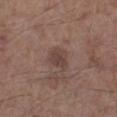No biopsy was performed on this lesion — it was imaged during a full skin examination and was not determined to be concerning.
The patient is a male aged 58–62.
A close-up tile cropped from a whole-body skin photograph, about 15 mm across.
The tile uses white-light illumination.
Longest diameter approximately 2.5 mm.
From the left lower leg.